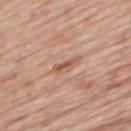The lesion was photographed on a routine skin check and not biopsied; there is no pathology result.